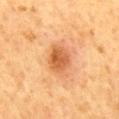This lesion was catalogued during total-body skin photography and was not selected for biopsy.
The patient is a male approximately 60 years of age.
Located on the mid back.
Automated image analysis of the tile measured an area of roughly 8 mm² and an outline eccentricity of about 0.55 (0 = round, 1 = elongated). The analysis additionally found a classifier nevus-likeness of about 85/100 and a detector confidence of about 100 out of 100 that the crop contains a lesion.
Longest diameter approximately 3.5 mm.
This is a cross-polarized tile.
This image is a 15 mm lesion crop taken from a total-body photograph.